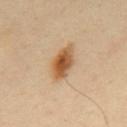body site: the mid back | illumination: cross-polarized illumination | acquisition: ~15 mm tile from a whole-body skin photo | size: ≈5 mm | automated lesion analysis: a lesion area of about 8.5 mm² and an outline eccentricity of about 0.85 (0 = round, 1 = elongated); border irregularity of about 2 on a 0–10 scale, internal color variation of about 6.5 on a 0–10 scale, and radial color variation of about 2; an automated nevus-likeness rating near 100 out of 100 and lesion-presence confidence of about 100/100 | subject: male, roughly 50 years of age.